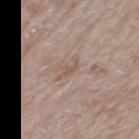biopsy_status: not biopsied; imaged during a skin examination
lesion_size:
  long_diameter_mm_approx: 3.0
image:
  source: total-body photography crop
  field_of_view_mm: 15
lighting: white-light
patient:
  sex: female
  age_approx: 75
site: left thigh